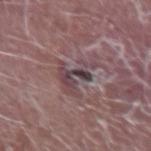<record>
  <biopsy_status>not biopsied; imaged during a skin examination</biopsy_status>
  <image>
    <source>total-body photography crop</source>
    <field_of_view_mm>15</field_of_view_mm>
  </image>
  <automated_metrics>
    <cielab_L>43</cielab_L>
    <cielab_a>17</cielab_a>
    <cielab_b>13</cielab_b>
    <vs_skin_darker_L>9.0</vs_skin_darker_L>
    <vs_skin_contrast_norm>7.5</vs_skin_contrast_norm>
  </automated_metrics>
  <patient>
    <sex>male</sex>
    <age_approx>65</age_approx>
  </patient>
  <site>right forearm</site>
</record>